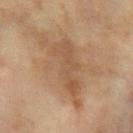anatomic site: the left forearm | automated metrics: border irregularity of about 7.5 on a 0–10 scale; a detector confidence of about 100 out of 100 that the crop contains a lesion | diameter: ~8 mm (longest diameter) | patient: female, in their 60s | acquisition: ~15 mm crop, total-body skin-cancer survey | tile lighting: cross-polarized.